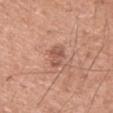Case summary:
• notes — no biopsy performed (imaged during a skin exam)
• site — the left upper arm
• subject — male, aged 53 to 57
• image — ~15 mm tile from a whole-body skin photo